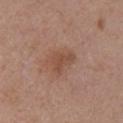Acquisition and patient details: A close-up tile cropped from a whole-body skin photograph, about 15 mm across. The subject is a female approximately 55 years of age. The lesion is on the arm. The recorded lesion diameter is about 3 mm. Captured under white-light illumination.